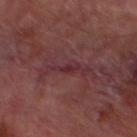Impression: No biopsy was performed on this lesion — it was imaged during a full skin examination and was not determined to be concerning. Image and clinical context: Longest diameter approximately 3 mm. A 15 mm crop from a total-body photograph taken for skin-cancer surveillance. Captured under cross-polarized illumination. A male subject approximately 70 years of age. The lesion is on the left lower leg. The total-body-photography lesion software estimated a lesion color around L≈29 a*≈24 b*≈15 in CIELAB and a normalized border contrast of about 6. The analysis additionally found a border-irregularity rating of about 4/10, a color-variation rating of about 0/10, and a peripheral color-asymmetry measure near 0.